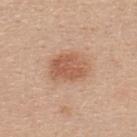Q: Is there a histopathology result?
A: no biopsy performed (imaged during a skin exam)